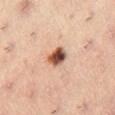Captured during whole-body skin photography for melanoma surveillance; the lesion was not biopsied. On the right thigh. The tile uses cross-polarized illumination. The total-body-photography lesion software estimated a lesion area of about 5.5 mm², an eccentricity of roughly 0.5, and a symmetry-axis asymmetry near 0.2. It also reported a border-irregularity index near 2/10, a within-lesion color-variation index near 8/10, and a peripheral color-asymmetry measure near 2.5. The software also gave a classifier nevus-likeness of about 100/100 and a detector confidence of about 100 out of 100 that the crop contains a lesion. A male patient about 55 years old. About 3 mm across. A close-up tile cropped from a whole-body skin photograph, about 15 mm across.